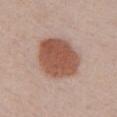Clinical impression: No biopsy was performed on this lesion — it was imaged during a full skin examination and was not determined to be concerning. Context: A lesion tile, about 15 mm wide, cut from a 3D total-body photograph. Located on the chest. The patient is a female in their 40s.